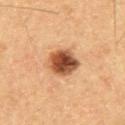Assessment: No biopsy was performed on this lesion — it was imaged during a full skin examination and was not determined to be concerning. Acquisition and patient details: A male patient, aged 58–62. A close-up tile cropped from a whole-body skin photograph, about 15 mm across. The tile uses cross-polarized illumination. An algorithmic analysis of the crop reported an eccentricity of roughly 0.6 and two-axis asymmetry of about 0.1. The analysis additionally found a border-irregularity index near 1/10, internal color variation of about 8 on a 0–10 scale, and peripheral color asymmetry of about 2.5. It also reported a classifier nevus-likeness of about 100/100 and a lesion-detection confidence of about 100/100.